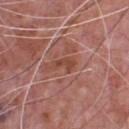Impression:
The lesion was tiled from a total-body skin photograph and was not biopsied.
Clinical summary:
The tile uses white-light illumination. A 15 mm crop from a total-body photograph taken for skin-cancer surveillance. From the chest. The subject is a male in their 70s.